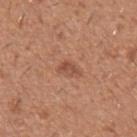* image — ~15 mm tile from a whole-body skin photo
* location — the left forearm
* subject — male, aged around 40
* size — ≈2.5 mm
* TBP lesion metrics — a border-irregularity index near 3.5/10 and a peripheral color-asymmetry measure near 1